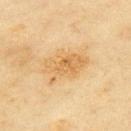The lesion was tiled from a total-body skin photograph and was not biopsied.
A female patient aged approximately 60.
A region of skin cropped from a whole-body photographic capture, roughly 15 mm wide.
The lesion is located on the back.
The recorded lesion diameter is about 5.5 mm.
The tile uses cross-polarized illumination.